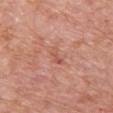notes: total-body-photography surveillance lesion; no biopsy | lesion diameter: ≈2.5 mm | location: the chest | lighting: white-light illumination | automated metrics: an area of roughly 2.5 mm²; a peripheral color-asymmetry measure near 0; an automated nevus-likeness rating near 0 out of 100 and a lesion-detection confidence of about 100/100 | patient: female, approximately 65 years of age | image: total-body-photography crop, ~15 mm field of view.